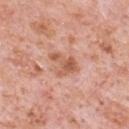The lesion was photographed on a routine skin check and not biopsied; there is no pathology result. A male patient, approximately 80 years of age. A 15 mm close-up tile from a total-body photography series done for melanoma screening. Captured under white-light illumination. From the chest.